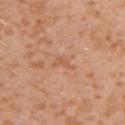<tbp_lesion>
<biopsy_status>not biopsied; imaged during a skin examination</biopsy_status>
<patient>
  <sex>female</sex>
  <age_approx>30</age_approx>
</patient>
<image>
  <source>total-body photography crop</source>
  <field_of_view_mm>15</field_of_view_mm>
</image>
<site>right upper arm</site>
</tbp_lesion>